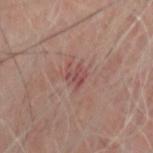workup: total-body-photography surveillance lesion; no biopsy | tile lighting: cross-polarized illumination | subject: male, approximately 55 years of age | lesion size: ≈2.5 mm | location: the head or neck | image source: total-body-photography crop, ~15 mm field of view.